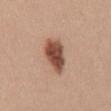Impression:
No biopsy was performed on this lesion — it was imaged during a full skin examination and was not determined to be concerning.
Background:
A female patient, in their mid- to late 30s. A close-up tile cropped from a whole-body skin photograph, about 15 mm across. Located on the back. Captured under white-light illumination.